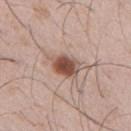Assessment: Imaged during a routine full-body skin examination; the lesion was not biopsied and no histopathology is available. Context: The recorded lesion diameter is about 3.5 mm. The patient is a male approximately 50 years of age. This image is a 15 mm lesion crop taken from a total-body photograph. Captured under white-light illumination. From the right upper arm.